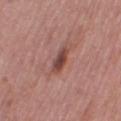Q: Was this lesion biopsied?
A: no biopsy performed (imaged during a skin exam)
Q: Lesion size?
A: ≈3 mm
Q: What are the patient's age and sex?
A: female, aged approximately 50
Q: What did automated image analysis measure?
A: border irregularity of about 2.5 on a 0–10 scale, internal color variation of about 3.5 on a 0–10 scale, and radial color variation of about 1
Q: Where on the body is the lesion?
A: the right thigh
Q: How was this image acquired?
A: ~15 mm crop, total-body skin-cancer survey
Q: What lighting was used for the tile?
A: white-light illumination